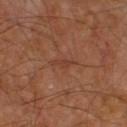Notes:
- body site · the upper back
- diameter · ≈3 mm
- lighting · cross-polarized
- image source · total-body-photography crop, ~15 mm field of view
- subject · male, in their mid-50s